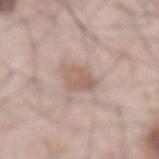workup: imaged on a skin check; not biopsied | automated lesion analysis: a shape eccentricity near 0.4 and a symmetry-axis asymmetry near 0.25; an average lesion color of about L≈59 a*≈18 b*≈24 (CIELAB) and a normalized border contrast of about 5.5 | acquisition: ~15 mm tile from a whole-body skin photo | lesion size: ≈3 mm | illumination: white-light illumination | body site: the front of the torso | patient: male, in their mid-60s.